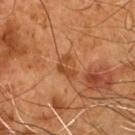Impression:
This lesion was catalogued during total-body skin photography and was not selected for biopsy.
Image and clinical context:
An algorithmic analysis of the crop reported a border-irregularity index near 5/10, a within-lesion color-variation index near 3.5/10, and radial color variation of about 1. Located on the front of the torso. Imaged with cross-polarized lighting. A roughly 15 mm field-of-view crop from a total-body skin photograph. The subject is a male aged around 55. About 3 mm across.